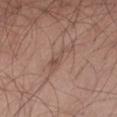Findings:
– workup · imaged on a skin check; not biopsied
– automated lesion analysis · a lesion color around L≈51 a*≈18 b*≈24 in CIELAB and a lesion–skin lightness drop of about 7
– tile lighting · white-light illumination
– diameter · about 4.5 mm
– subject · male, aged 53 to 57
– imaging modality · ~15 mm tile from a whole-body skin photo
– body site · the front of the torso